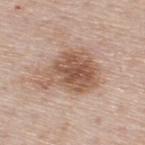<tbp_lesion>
  <biopsy_status>not biopsied; imaged during a skin examination</biopsy_status>
  <patient>
    <sex>male</sex>
    <age_approx>60</age_approx>
  </patient>
  <image>
    <source>total-body photography crop</source>
    <field_of_view_mm>15</field_of_view_mm>
  </image>
  <site>upper back</site>
  <lighting>white-light</lighting>
</tbp_lesion>